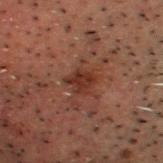Captured under cross-polarized illumination. The patient is a male aged 48–52. Longest diameter approximately 3 mm. The lesion is on the head or neck. Cropped from a whole-body photographic skin survey; the tile spans about 15 mm.